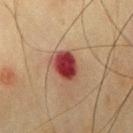workup = imaged on a skin check; not biopsied
TBP lesion metrics = border irregularity of about 2 on a 0–10 scale and a peripheral color-asymmetry measure near 1.5
anatomic site = the right upper arm
acquisition = 15 mm crop, total-body photography
patient = male, aged 73–77
lighting = cross-polarized